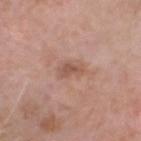Imaged with white-light lighting. Cropped from a whole-body photographic skin survey; the tile spans about 15 mm. Located on the head or neck. A male patient in their mid- to late 60s. The lesion's longest dimension is about 2.5 mm. An algorithmic analysis of the crop reported an area of roughly 3 mm² and an eccentricity of roughly 0.8. The software also gave a border-irregularity index near 3.5/10, a within-lesion color-variation index near 0.5/10, and a peripheral color-asymmetry measure near 0.